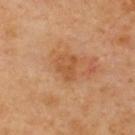The lesion was photographed on a routine skin check and not biopsied; there is no pathology result. On the back. A male patient aged around 70. The lesion-visualizer software estimated a border-irregularity rating of about 3/10 and a within-lesion color-variation index near 2.5/10. And it measured a nevus-likeness score of about 0/100 and a lesion-detection confidence of about 100/100. Measured at roughly 3 mm in maximum diameter. A roughly 15 mm field-of-view crop from a total-body skin photograph. Captured under cross-polarized illumination.